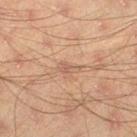follow-up = no biopsy performed (imaged during a skin exam) | illumination = cross-polarized | location = the left thigh | lesion size = about 2.5 mm | acquisition = total-body-photography crop, ~15 mm field of view | subject = male, aged 43 to 47.